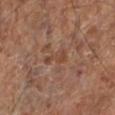The lesion was photographed on a routine skin check and not biopsied; there is no pathology result. Captured under cross-polarized illumination. The recorded lesion diameter is about 3.5 mm. A patient aged 63 to 67. A 15 mm close-up extracted from a 3D total-body photography capture. Automated tile analysis of the lesion measured a shape eccentricity near 0.85 and two-axis asymmetry of about 0.5. The analysis additionally found a border-irregularity index near 5/10 and peripheral color asymmetry of about 0.5. It also reported an automated nevus-likeness rating near 0 out of 100 and lesion-presence confidence of about 95/100. The lesion is on the leg.